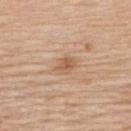notes: imaged on a skin check; not biopsied
site: the upper back
subject: female, aged 63–67
lesion size: ≈2.5 mm
automated lesion analysis: a footprint of about 4.5 mm² and a shape-asymmetry score of about 0.3 (0 = symmetric); a lesion color around L≈59 a*≈20 b*≈33 in CIELAB, a lesion–skin lightness drop of about 9, and a normalized lesion–skin contrast near 6.5; border irregularity of about 2.5 on a 0–10 scale, internal color variation of about 2.5 on a 0–10 scale, and peripheral color asymmetry of about 1
imaging modality: ~15 mm tile from a whole-body skin photo